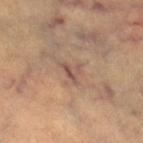Part of a total-body skin-imaging series; this lesion was reviewed on a skin check and was not flagged for biopsy. This is a cross-polarized tile. A female patient, approximately 30 years of age. Located on the right thigh. Longest diameter approximately 2.5 mm. Cropped from a whole-body photographic skin survey; the tile spans about 15 mm.